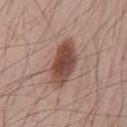This lesion was catalogued during total-body skin photography and was not selected for biopsy. A roughly 15 mm field-of-view crop from a total-body skin photograph. On the chest. This is a white-light tile. A male subject, aged 63–67. Approximately 6.5 mm at its widest.